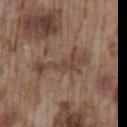Assessment: The lesion was photographed on a routine skin check and not biopsied; there is no pathology result. Acquisition and patient details: Cropped from a whole-body photographic skin survey; the tile spans about 15 mm. Located on the lower back. Imaged with white-light lighting. The recorded lesion diameter is about 7 mm. A male patient, about 75 years old.